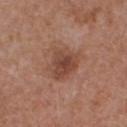notes = imaged on a skin check; not biopsied
anatomic site = the front of the torso
imaging modality = ~15 mm crop, total-body skin-cancer survey
patient = male, aged approximately 55
tile lighting = white-light